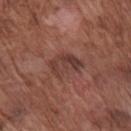biopsy_status: not biopsied; imaged during a skin examination
automated_metrics:
  eccentricity: 0.75
  shape_asymmetry: 0.35
  cielab_L: 39
  cielab_a: 21
  cielab_b: 24
  vs_skin_darker_L: 8.0
  vs_skin_contrast_norm: 6.5
site: arm
lesion_size:
  long_diameter_mm_approx: 4.0
patient:
  sex: male
  age_approx: 75
lighting: white-light
image:
  source: total-body photography crop
  field_of_view_mm: 15